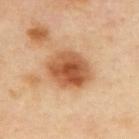Impression:
Imaged during a routine full-body skin examination; the lesion was not biopsied and no histopathology is available.